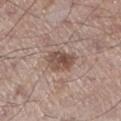Case summary:
• workup — total-body-photography surveillance lesion; no biopsy
• image — ~15 mm tile from a whole-body skin photo
• location — the left lower leg
• subject — male, in their mid-50s